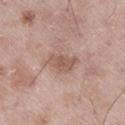{
  "biopsy_status": "not biopsied; imaged during a skin examination",
  "image": {
    "source": "total-body photography crop",
    "field_of_view_mm": 15
  },
  "lesion_size": {
    "long_diameter_mm_approx": 4.0
  },
  "site": "leg",
  "patient": {
    "sex": "male",
    "age_approx": 50
  }
}